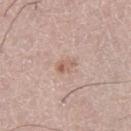  biopsy_status: not biopsied; imaged during a skin examination
  lesion_size:
    long_diameter_mm_approx: 2.5
  image:
    source: total-body photography crop
    field_of_view_mm: 15
  lighting: white-light
  site: left thigh
  patient:
    sex: male
    age_approx: 65